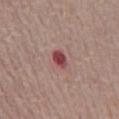Assessment: Imaged during a routine full-body skin examination; the lesion was not biopsied and no histopathology is available. Image and clinical context: Imaged with white-light lighting. A male subject, aged approximately 75. The lesion's longest dimension is about 2.5 mm. The lesion is located on the front of the torso. A roughly 15 mm field-of-view crop from a total-body skin photograph.